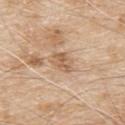The lesion was photographed on a routine skin check and not biopsied; there is no pathology result. Cropped from a total-body skin-imaging series; the visible field is about 15 mm. The lesion is located on the upper back. About 3 mm across. The lesion-visualizer software estimated an average lesion color of about L≈60 a*≈18 b*≈33 (CIELAB), roughly 9 lightness units darker than nearby skin, and a normalized lesion–skin contrast near 6.5. The software also gave a color-variation rating of about 4/10 and peripheral color asymmetry of about 1.5. And it measured lesion-presence confidence of about 100/100. A male patient in their 80s.